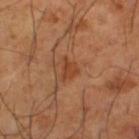Impression:
Imaged during a routine full-body skin examination; the lesion was not biopsied and no histopathology is available.
Clinical summary:
The lesion is located on the left thigh. About 3 mm across. Imaged with cross-polarized lighting. Automated tile analysis of the lesion measured an automated nevus-likeness rating near 65 out of 100. A region of skin cropped from a whole-body photographic capture, roughly 15 mm wide. A male patient aged 63–67.